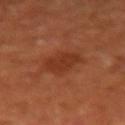Clinical impression:
This lesion was catalogued during total-body skin photography and was not selected for biopsy.
Context:
Cropped from a total-body skin-imaging series; the visible field is about 15 mm. The lesion is located on the right forearm. A female patient aged around 65.